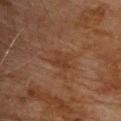No biopsy was performed on this lesion — it was imaged during a full skin examination and was not determined to be concerning. The patient is a male aged 73–77. Imaged with cross-polarized lighting. Approximately 3 mm at its widest. The total-body-photography lesion software estimated a border-irregularity index near 3.5/10, a within-lesion color-variation index near 1.5/10, and peripheral color asymmetry of about 0.5. Cropped from a total-body skin-imaging series; the visible field is about 15 mm. Located on the chest.